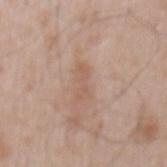Q: Is there a histopathology result?
A: no biopsy performed (imaged during a skin exam)
Q: How was this image acquired?
A: 15 mm crop, total-body photography
Q: How was the tile lit?
A: white-light illumination
Q: What is the lesion's diameter?
A: ~3.5 mm (longest diameter)
Q: Who is the patient?
A: male, aged approximately 50
Q: Where on the body is the lesion?
A: the mid back